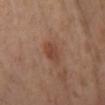Q: Was this lesion biopsied?
A: imaged on a skin check; not biopsied
Q: Patient demographics?
A: female, in their mid-50s
Q: Lesion size?
A: ~3 mm (longest diameter)
Q: What is the imaging modality?
A: ~15 mm crop, total-body skin-cancer survey
Q: Automated lesion metrics?
A: an area of roughly 5 mm² and two-axis asymmetry of about 0.3; a nevus-likeness score of about 20/100
Q: Where on the body is the lesion?
A: the left lower leg